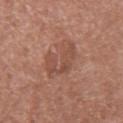Image and clinical context: Cropped from a whole-body photographic skin survey; the tile spans about 15 mm. An algorithmic analysis of the crop reported border irregularity of about 9 on a 0–10 scale and a peripheral color-asymmetry measure near 0.5. The analysis additionally found a lesion-detection confidence of about 100/100. From the front of the torso. This is a white-light tile. The subject is a male aged approximately 75.